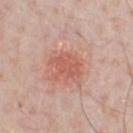Impression:
This lesion was catalogued during total-body skin photography and was not selected for biopsy.
Context:
Captured under white-light illumination. A male subject, aged 58–62. Approximately 3.5 mm at its widest. The lesion is on the chest. This image is a 15 mm lesion crop taken from a total-body photograph.